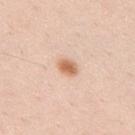workup: catalogued during a skin exam; not biopsied | image source: total-body-photography crop, ~15 mm field of view | site: the upper back | lighting: white-light | automated metrics: an area of roughly 4 mm², a shape eccentricity near 0.75, and a shape-asymmetry score of about 0.25 (0 = symmetric); about 13 CIELAB-L* units darker than the surrounding skin | patient: male, aged around 35 | size: ≈2.5 mm.